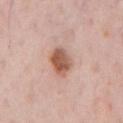No biopsy was performed on this lesion — it was imaged during a full skin examination and was not determined to be concerning. The lesion is on the chest. A roughly 15 mm field-of-view crop from a total-body skin photograph. A male patient, aged 63 to 67. This is a white-light tile. Longest diameter approximately 4 mm.